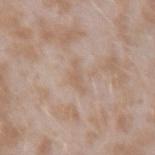Q: Was this lesion biopsied?
A: imaged on a skin check; not biopsied
Q: What kind of image is this?
A: ~15 mm tile from a whole-body skin photo
Q: How was the tile lit?
A: white-light illumination
Q: Patient demographics?
A: female, about 25 years old
Q: Where on the body is the lesion?
A: the arm